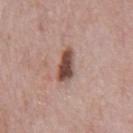Case summary:
- body site: the chest
- image source: total-body-photography crop, ~15 mm field of view
- size: ≈4 mm
- subject: male, about 70 years old
- illumination: white-light